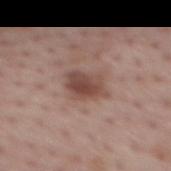Part of a total-body skin-imaging series; this lesion was reviewed on a skin check and was not flagged for biopsy. About 4 mm across. The tile uses white-light illumination. A male patient, aged around 75. A 15 mm close-up tile from a total-body photography series done for melanoma screening. On the back.